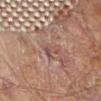Captured during whole-body skin photography for melanoma surveillance; the lesion was not biopsied. The lesion is located on the right forearm. About 2.5 mm across. Cropped from a total-body skin-imaging series; the visible field is about 15 mm. Captured under cross-polarized illumination. A male patient, aged 83 or over.